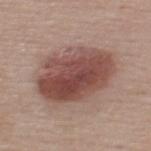A female patient about 55 years old. From the upper back. A 15 mm close-up tile from a total-body photography series done for melanoma screening. The lesion was biopsied, and histopathology showed a congenital melanocytic nevus, classified as a benign lesion.